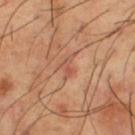About 2.5 mm across. The lesion is located on the left thigh. A 15 mm close-up tile from a total-body photography series done for melanoma screening. The patient is a male roughly 65 years of age.